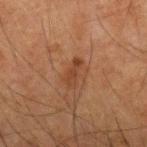Acquisition and patient details: Located on the right forearm. The subject is a male aged approximately 65. A 15 mm close-up tile from a total-body photography series done for melanoma screening.